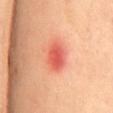Q: Was a biopsy performed?
A: no biopsy performed (imaged during a skin exam)
Q: Illumination type?
A: cross-polarized illumination
Q: How was this image acquired?
A: total-body-photography crop, ~15 mm field of view
Q: Automated lesion metrics?
A: border irregularity of about 1.5 on a 0–10 scale and peripheral color asymmetry of about 1; a classifier nevus-likeness of about 0/100 and lesion-presence confidence of about 100/100
Q: What is the lesion's diameter?
A: ~3.5 mm (longest diameter)
Q: What is the anatomic site?
A: the mid back
Q: What are the patient's age and sex?
A: female, aged 38 to 42